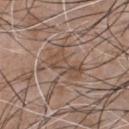<lesion>
  <patient>
    <sex>male</sex>
    <age_approx>50</age_approx>
  </patient>
  <lighting>white-light</lighting>
  <site>chest</site>
  <image>
    <source>total-body photography crop</source>
    <field_of_view_mm>15</field_of_view_mm>
  </image>
</lesion>